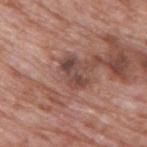follow-up — no biopsy performed (imaged during a skin exam)
location — the upper back
patient — male, in their 70s
imaging modality — 15 mm crop, total-body photography
diameter — ≈3.5 mm
tile lighting — white-light